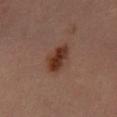Part of a total-body skin-imaging series; this lesion was reviewed on a skin check and was not flagged for biopsy.